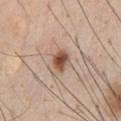{
  "biopsy_status": "not biopsied; imaged during a skin examination",
  "image": {
    "source": "total-body photography crop",
    "field_of_view_mm": 15
  },
  "automated_metrics": {
    "eccentricity": 0.65,
    "border_irregularity_0_10": 2.0,
    "color_variation_0_10": 4.5,
    "peripheral_color_asymmetry": 1.5
  },
  "patient": {
    "sex": "male",
    "age_approx": 60
  },
  "lighting": "white-light",
  "lesion_size": {
    "long_diameter_mm_approx": 2.5
  },
  "site": "chest"
}